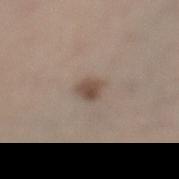This lesion was catalogued during total-body skin photography and was not selected for biopsy.
The lesion is on the left lower leg.
A 15 mm close-up tile from a total-body photography series done for melanoma screening.
The recorded lesion diameter is about 2.5 mm.
Captured under white-light illumination.
A female patient aged 38–42.
The total-body-photography lesion software estimated a lesion area of about 3.5 mm², a shape eccentricity near 0.75, and a shape-asymmetry score of about 0.25 (0 = symmetric).